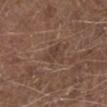Part of a total-body skin-imaging series; this lesion was reviewed on a skin check and was not flagged for biopsy. A 15 mm crop from a total-body photograph taken for skin-cancer surveillance. The subject is a male aged 73–77. The lesion is located on the left lower leg. This is a white-light tile. Approximately 3 mm at its widest.